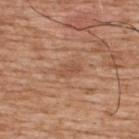* follow-up: catalogued during a skin exam; not biopsied
* diameter: ~2.5 mm (longest diameter)
* imaging modality: 15 mm crop, total-body photography
* location: the upper back
* lighting: white-light
* TBP lesion metrics: a footprint of about 3 mm² and a shape eccentricity near 0.85
* patient: male, approximately 60 years of age